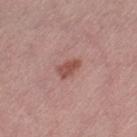notes: catalogued during a skin exam; not biopsied
body site: the left thigh
subject: female, roughly 40 years of age
image-analysis metrics: a border-irregularity index near 2.5/10 and internal color variation of about 2 on a 0–10 scale; a lesion-detection confidence of about 100/100
illumination: white-light illumination
image source: ~15 mm crop, total-body skin-cancer survey
lesion size: about 3 mm